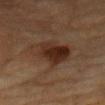No biopsy was performed on this lesion — it was imaged during a full skin examination and was not determined to be concerning. A male subject, aged 83 or older. From the chest. A lesion tile, about 15 mm wide, cut from a 3D total-body photograph.